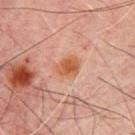Imaged during a routine full-body skin examination; the lesion was not biopsied and no histopathology is available. Cropped from a whole-body photographic skin survey; the tile spans about 15 mm. The lesion's longest dimension is about 3.5 mm. The total-body-photography lesion software estimated a mean CIELAB color near L≈47 a*≈22 b*≈31, roughly 8 lightness units darker than nearby skin, and a lesion-to-skin contrast of about 8.5 (normalized; higher = more distinct). It also reported a border-irregularity index near 2.5/10, a within-lesion color-variation index near 3.5/10, and peripheral color asymmetry of about 1. A male subject, aged 68 to 72. This is a cross-polarized tile. Located on the mid back.